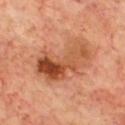| field | value |
|---|---|
| follow-up | catalogued during a skin exam; not biopsied |
| lighting | cross-polarized illumination |
| size | ~7 mm (longest diameter) |
| subject | male, approximately 70 years of age |
| acquisition | total-body-photography crop, ~15 mm field of view |
| location | the front of the torso |